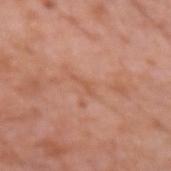{
  "biopsy_status": "not biopsied; imaged during a skin examination",
  "lighting": "white-light",
  "site": "arm",
  "patient": {
    "sex": "male",
    "age_approx": 75
  },
  "image": {
    "source": "total-body photography crop",
    "field_of_view_mm": 15
  },
  "lesion_size": {
    "long_diameter_mm_approx": 2.5
  }
}